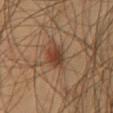This lesion was catalogued during total-body skin photography and was not selected for biopsy. The patient is a male roughly 45 years of age. The total-body-photography lesion software estimated an outline eccentricity of about 0.55 (0 = round, 1 = elongated) and two-axis asymmetry of about 0.2. The software also gave a lesion color around L≈40 a*≈20 b*≈30 in CIELAB. The analysis additionally found a within-lesion color-variation index near 6/10 and a peripheral color-asymmetry measure near 2.5. The analysis additionally found an automated nevus-likeness rating near 85 out of 100. The lesion is located on the chest. Approximately 3.5 mm at its widest. A roughly 15 mm field-of-view crop from a total-body skin photograph. Captured under cross-polarized illumination.